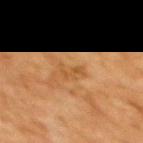Recorded during total-body skin imaging; not selected for excision or biopsy.
A roughly 15 mm field-of-view crop from a total-body skin photograph.
Longest diameter approximately 3 mm.
A female subject, roughly 55 years of age.
The tile uses cross-polarized illumination.
The lesion is on the upper back.
The total-body-photography lesion software estimated an area of roughly 3 mm², a shape eccentricity near 0.95, and two-axis asymmetry of about 0.45.